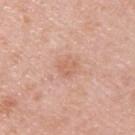Imaged during a routine full-body skin examination; the lesion was not biopsied and no histopathology is available. A close-up tile cropped from a whole-body skin photograph, about 15 mm across. This is a white-light tile. The total-body-photography lesion software estimated a classifier nevus-likeness of about 0/100 and a lesion-detection confidence of about 100/100. The lesion is located on the upper back. A male subject, approximately 45 years of age.